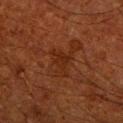Context:
Cropped from a total-body skin-imaging series; the visible field is about 15 mm. A male patient in their 60s. From the right upper arm. Longest diameter approximately 3 mm. Captured under cross-polarized illumination.